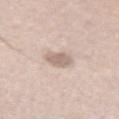Assessment:
No biopsy was performed on this lesion — it was imaged during a full skin examination and was not determined to be concerning.
Acquisition and patient details:
The lesion is on the left upper arm. A male patient roughly 45 years of age. A 15 mm crop from a total-body photograph taken for skin-cancer surveillance. This is a white-light tile. Approximately 3 mm at its widest.